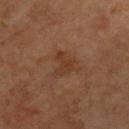Impression: The lesion was photographed on a routine skin check and not biopsied; there is no pathology result. Clinical summary: Captured under cross-polarized illumination. Automated image analysis of the tile measured a classifier nevus-likeness of about 0/100 and lesion-presence confidence of about 100/100. The lesion is located on the chest. A region of skin cropped from a whole-body photographic capture, roughly 15 mm wide. Measured at roughly 3 mm in maximum diameter. A male patient, about 60 years old.